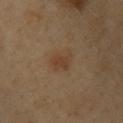Captured during whole-body skin photography for melanoma surveillance; the lesion was not biopsied. This is a cross-polarized tile. A male patient aged 38 to 42. About 3.5 mm across. Automated tile analysis of the lesion measured an area of roughly 5 mm² and an outline eccentricity of about 0.8 (0 = round, 1 = elongated). The software also gave a lesion color around L≈40 a*≈17 b*≈29 in CIELAB, roughly 6 lightness units darker than nearby skin, and a normalized border contrast of about 6. The software also gave a nevus-likeness score of about 50/100 and lesion-presence confidence of about 100/100. Located on the left upper arm. A 15 mm close-up extracted from a 3D total-body photography capture.